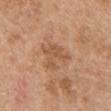Clinical impression:
Part of a total-body skin-imaging series; this lesion was reviewed on a skin check and was not flagged for biopsy.
Image and clinical context:
Measured at roughly 4.5 mm in maximum diameter. This is a white-light tile. The patient is a male aged around 50. Located on the chest. A region of skin cropped from a whole-body photographic capture, roughly 15 mm wide. The total-body-photography lesion software estimated a lesion color around L≈54 a*≈21 b*≈34 in CIELAB, a lesion–skin lightness drop of about 8, and a normalized border contrast of about 5.5. The software also gave an automated nevus-likeness rating near 0 out of 100 and a lesion-detection confidence of about 100/100.